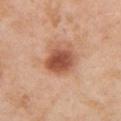{
  "biopsy_status": "not biopsied; imaged during a skin examination",
  "automated_metrics": {
    "area_mm2_approx": 11.0,
    "eccentricity": 0.45,
    "border_irregularity_0_10": 1.5,
    "color_variation_0_10": 4.5,
    "peripheral_color_asymmetry": 1.5
  },
  "patient": {
    "sex": "female",
    "age_approx": 40
  },
  "image": {
    "source": "total-body photography crop",
    "field_of_view_mm": 15
  },
  "site": "left upper arm",
  "lesion_size": {
    "long_diameter_mm_approx": 4.0
  },
  "lighting": "white-light"
}